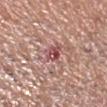{
  "biopsy_status": "not biopsied; imaged during a skin examination",
  "lesion_size": {
    "long_diameter_mm_approx": 3.0
  },
  "site": "head or neck",
  "image": {
    "source": "total-body photography crop",
    "field_of_view_mm": 15
  },
  "patient": {
    "sex": "male",
    "age_approx": 60
  },
  "lighting": "white-light"
}